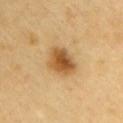Clinical impression:
Imaged during a routine full-body skin examination; the lesion was not biopsied and no histopathology is available.
Background:
On the chest. A male patient, in their 60s. The recorded lesion diameter is about 4 mm. A close-up tile cropped from a whole-body skin photograph, about 15 mm across.